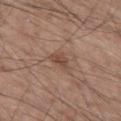{
  "biopsy_status": "not biopsied; imaged during a skin examination",
  "lesion_size": {
    "long_diameter_mm_approx": 3.0
  },
  "lighting": "white-light",
  "image": {
    "source": "total-body photography crop",
    "field_of_view_mm": 15
  },
  "patient": {
    "sex": "male",
    "age_approx": 60
  },
  "automated_metrics": {
    "cielab_L": 47,
    "cielab_a": 19,
    "cielab_b": 27,
    "vs_skin_darker_L": 9.0,
    "vs_skin_contrast_norm": 6.5,
    "border_irregularity_0_10": 2.5,
    "peripheral_color_asymmetry": 1.0
  },
  "site": "left thigh"
}